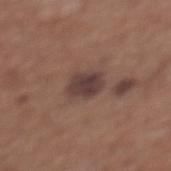Impression: No biopsy was performed on this lesion — it was imaged during a full skin examination and was not determined to be concerning. Background: This is a white-light tile. Automated image analysis of the tile measured a mean CIELAB color near L≈39 a*≈17 b*≈20, roughly 10 lightness units darker than nearby skin, and a normalized border contrast of about 9.5. And it measured a border-irregularity index near 2/10. On the upper back. The patient is a female in their mid- to late 60s. Approximately 3.5 mm at its widest. Cropped from a whole-body photographic skin survey; the tile spans about 15 mm.